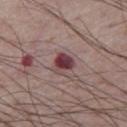Q: Was this lesion biopsied?
A: no biopsy performed (imaged during a skin exam)
Q: What did automated image analysis measure?
A: an area of roughly 6 mm², an eccentricity of roughly 0.5, and a symmetry-axis asymmetry near 0.2; radial color variation of about 2; a classifier nevus-likeness of about 0/100 and a detector confidence of about 100 out of 100 that the crop contains a lesion
Q: Lesion size?
A: ~3 mm (longest diameter)
Q: Lesion location?
A: the leg
Q: What kind of image is this?
A: 15 mm crop, total-body photography
Q: What are the patient's age and sex?
A: male, aged approximately 75